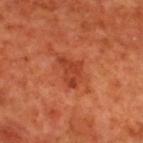follow-up: total-body-photography surveillance lesion; no biopsy | patient: male, about 70 years old | tile lighting: cross-polarized illumination | automated metrics: an area of roughly 7 mm², an outline eccentricity of about 0.75 (0 = round, 1 = elongated), and two-axis asymmetry of about 0.45 | site: the upper back | acquisition: 15 mm crop, total-body photography.